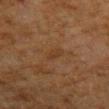| field | value |
|---|---|
| notes | total-body-photography surveillance lesion; no biopsy |
| location | the left upper arm |
| subject | male, approximately 80 years of age |
| lighting | cross-polarized illumination |
| size | about 3 mm |
| image source | ~15 mm crop, total-body skin-cancer survey |